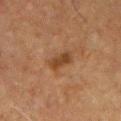notes: no biopsy performed (imaged during a skin exam); site: the chest; image source: total-body-photography crop, ~15 mm field of view; tile lighting: cross-polarized illumination; subject: male, in their mid-60s; diameter: about 3 mm.